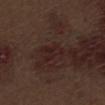Recorded during total-body skin imaging; not selected for excision or biopsy. The lesion-visualizer software estimated a lesion area of about 5 mm². The software also gave a nevus-likeness score of about 0/100 and lesion-presence confidence of about 100/100. Captured under white-light illumination. Cropped from a total-body skin-imaging series; the visible field is about 15 mm. Approximately 3 mm at its widest. A male subject aged 68–72. The lesion is located on the abdomen.